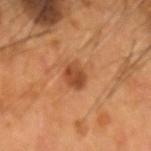<tbp_lesion>
<lesion_size>
  <long_diameter_mm_approx>3.0</long_diameter_mm_approx>
</lesion_size>
<patient>
  <sex>male</sex>
  <age_approx>55</age_approx>
</patient>
<automated_metrics>
  <cielab_L>43</cielab_L>
  <cielab_a>24</cielab_a>
  <cielab_b>34</cielab_b>
  <vs_skin_darker_L>10.0</vs_skin_darker_L>
  <vs_skin_contrast_norm>8.0</vs_skin_contrast_norm>
</automated_metrics>
<site>head or neck</site>
<image>
  <source>total-body photography crop</source>
  <field_of_view_mm>15</field_of_view_mm>
</image>
</tbp_lesion>